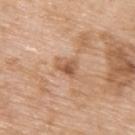Assessment: The lesion was tiled from a total-body skin photograph and was not biopsied. Clinical summary: A roughly 15 mm field-of-view crop from a total-body skin photograph. Located on the right upper arm. The total-body-photography lesion software estimated roughly 11 lightness units darker than nearby skin and a normalized lesion–skin contrast near 7.5. A female subject aged 73–77.